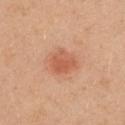Q: Was this lesion biopsied?
A: total-body-photography surveillance lesion; no biopsy